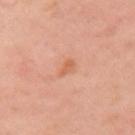<record>
<biopsy_status>not biopsied; imaged during a skin examination</biopsy_status>
<image>
  <source>total-body photography crop</source>
  <field_of_view_mm>15</field_of_view_mm>
</image>
<patient>
  <sex>female</sex>
  <age_approx>55</age_approx>
</patient>
<lesion_size>
  <long_diameter_mm_approx>2.5</long_diameter_mm_approx>
</lesion_size>
<lighting>cross-polarized</lighting>
<site>left upper arm</site>
</record>